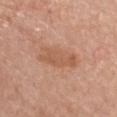notes: no biopsy performed (imaged during a skin exam) | subject: female, in their mid- to late 60s | TBP lesion metrics: a footprint of about 8.5 mm², an outline eccentricity of about 0.9 (0 = round, 1 = elongated), and two-axis asymmetry of about 0.3; a color-variation rating of about 2/10 and radial color variation of about 0.5; a classifier nevus-likeness of about 5/100 and lesion-presence confidence of about 100/100 | imaging modality: 15 mm crop, total-body photography | lighting: white-light illumination | location: the chest.